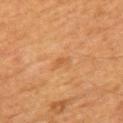notes: total-body-photography surveillance lesion; no biopsy
image-analysis metrics: a lesion color around L≈52 a*≈24 b*≈40 in CIELAB and a normalized lesion–skin contrast near 5
tile lighting: cross-polarized
patient: male, aged 58–62
image source: total-body-photography crop, ~15 mm field of view
body site: the mid back